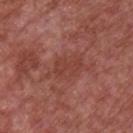workup: catalogued during a skin exam; not biopsied | image-analysis metrics: a lesion area of about 5 mm², an outline eccentricity of about 0.85 (0 = round, 1 = elongated), and a symmetry-axis asymmetry near 0.45; a mean CIELAB color near L≈41 a*≈26 b*≈27, about 6 CIELAB-L* units darker than the surrounding skin, and a normalized lesion–skin contrast near 5; a nevus-likeness score of about 0/100 and lesion-presence confidence of about 100/100 | image source: ~15 mm tile from a whole-body skin photo | size: about 3.5 mm | illumination: white-light illumination | anatomic site: the chest | subject: male, roughly 65 years of age.